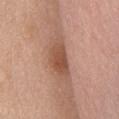Q: How large is the lesion?
A: ~5.5 mm (longest diameter)
Q: How was this image acquired?
A: ~15 mm tile from a whole-body skin photo
Q: How was the tile lit?
A: white-light
Q: Patient demographics?
A: female, aged 58–62
Q: What is the anatomic site?
A: the front of the torso